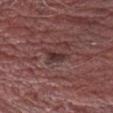Findings:
* follow-up — total-body-photography surveillance lesion; no biopsy
* diameter — ≈3 mm
* image source — ~15 mm tile from a whole-body skin photo
* automated lesion analysis — an area of roughly 3.5 mm², an eccentricity of roughly 0.85, and a shape-asymmetry score of about 0.3 (0 = symmetric); a nevus-likeness score of about 0/100 and a detector confidence of about 90 out of 100 that the crop contains a lesion
* patient — male, approximately 25 years of age
* anatomic site — the front of the torso